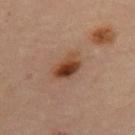<record>
  <biopsy_status>not biopsied; imaged during a skin examination</biopsy_status>
  <image>
    <source>total-body photography crop</source>
    <field_of_view_mm>15</field_of_view_mm>
  </image>
  <lighting>cross-polarized</lighting>
  <site>right thigh</site>
  <patient>
    <sex>female</sex>
    <age_approx>70</age_approx>
  </patient>
  <lesion_size>
    <long_diameter_mm_approx>4.0</long_diameter_mm_approx>
  </lesion_size>
  <automated_metrics>
    <area_mm2_approx>8.0</area_mm2_approx>
    <eccentricity>0.8</eccentricity>
    <color_variation_0_10>8.0</color_variation_0_10>
    <peripheral_color_asymmetry>2.5</peripheral_color_asymmetry>
    <nevus_likeness_0_100>100</nevus_likeness_0_100>
    <lesion_detection_confidence_0_100>100</lesion_detection_confidence_0_100>
  </automated_metrics>
</record>